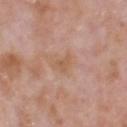biopsy_status: not biopsied; imaged during a skin examination
patient:
  sex: male
  age_approx: 70
lesion_size:
  long_diameter_mm_approx: 3.0
image:
  source: total-body photography crop
  field_of_view_mm: 15
site: head or neck